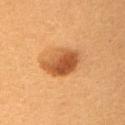Case summary:
- subject: female, aged around 30
- site: the left upper arm
- automated metrics: an area of roughly 14 mm², a shape eccentricity near 0.65, and a symmetry-axis asymmetry near 0.15; a classifier nevus-likeness of about 95/100
- imaging modality: ~15 mm crop, total-body skin-cancer survey